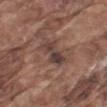Clinical impression:
The lesion was photographed on a routine skin check and not biopsied; there is no pathology result.
Clinical summary:
Located on the left upper arm. The lesion's longest dimension is about 4.5 mm. A 15 mm close-up extracted from a 3D total-body photography capture. The subject is a male about 75 years old. Captured under white-light illumination.